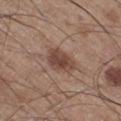Assessment: Recorded during total-body skin imaging; not selected for excision or biopsy.